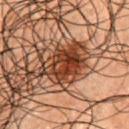Assessment: No biopsy was performed on this lesion — it was imaged during a full skin examination and was not determined to be concerning. Background: A male patient, aged approximately 50. Imaged with cross-polarized lighting. A 15 mm close-up extracted from a 3D total-body photography capture. The lesion is located on the front of the torso.